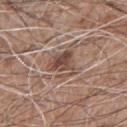Assessment:
Recorded during total-body skin imaging; not selected for excision or biopsy.